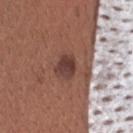  biopsy_status: not biopsied; imaged during a skin examination
  patient:
    sex: male
    age_approx: 35
  site: head or neck
  image:
    source: total-body photography crop
    field_of_view_mm: 15